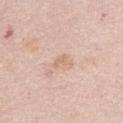Impression:
Recorded during total-body skin imaging; not selected for excision or biopsy.
Context:
The lesion is located on the abdomen. The tile uses white-light illumination. A region of skin cropped from a whole-body photographic capture, roughly 15 mm wide. A female patient in their mid- to late 60s. The recorded lesion diameter is about 2.5 mm.